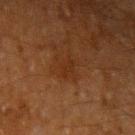{
  "biopsy_status": "not biopsied; imaged during a skin examination",
  "automated_metrics": {
    "cielab_L": 22,
    "cielab_a": 18,
    "cielab_b": 26,
    "vs_skin_contrast_norm": 5.5,
    "border_irregularity_0_10": 4.5,
    "color_variation_0_10": 0.0,
    "peripheral_color_asymmetry": 0.0
  },
  "patient": {
    "sex": "male",
    "age_approx": 60
  },
  "image": {
    "source": "total-body photography crop",
    "field_of_view_mm": 15
  },
  "site": "left upper arm",
  "lighting": "cross-polarized"
}